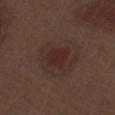  biopsy_status: not biopsied; imaged during a skin examination
  patient:
    sex: male
    age_approx: 70
  lighting: white-light
  site: right thigh
  image:
    source: total-body photography crop
    field_of_view_mm: 15